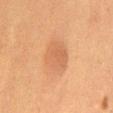Captured during whole-body skin photography for melanoma surveillance; the lesion was not biopsied. The lesion is located on the abdomen. A male subject, aged 48–52. A region of skin cropped from a whole-body photographic capture, roughly 15 mm wide.